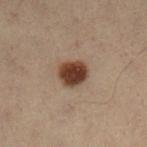– follow-up — total-body-photography surveillance lesion; no biopsy
– body site — the left lower leg
– acquisition — 15 mm crop, total-body photography
– lesion diameter — about 3.5 mm
– image-analysis metrics — an area of roughly 8 mm², an outline eccentricity of about 0.55 (0 = round, 1 = elongated), and a symmetry-axis asymmetry near 0.2; border irregularity of about 1.5 on a 0–10 scale, internal color variation of about 3.5 on a 0–10 scale, and a peripheral color-asymmetry measure near 1; a classifier nevus-likeness of about 100/100 and a lesion-detection confidence of about 100/100
– subject — male, aged around 55